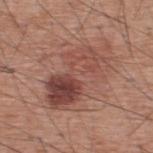workup = total-body-photography surveillance lesion; no biopsy
image = ~15 mm tile from a whole-body skin photo
illumination = white-light
patient = male, aged approximately 60
location = the upper back